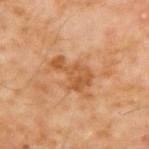<record>
  <biopsy_status>not biopsied; imaged during a skin examination</biopsy_status>
  <lesion_size>
    <long_diameter_mm_approx>5.0</long_diameter_mm_approx>
  </lesion_size>
  <lighting>cross-polarized</lighting>
  <automated_metrics>
    <area_mm2_approx>8.5</area_mm2_approx>
    <eccentricity>0.9</eccentricity>
    <shape_asymmetry>0.6</shape_asymmetry>
    <cielab_L>51</cielab_L>
    <cielab_a>23</cielab_a>
    <cielab_b>39</cielab_b>
    <vs_skin_darker_L>9.0</vs_skin_darker_L>
    <vs_skin_contrast_norm>7.0</vs_skin_contrast_norm>
    <border_irregularity_0_10>7.0</border_irregularity_0_10>
    <color_variation_0_10>3.0</color_variation_0_10>
    <peripheral_color_asymmetry>1.0</peripheral_color_asymmetry>
  </automated_metrics>
  <patient>
    <sex>male</sex>
    <age_approx>60</age_approx>
  </patient>
  <site>upper back</site>
  <image>
    <source>total-body photography crop</source>
    <field_of_view_mm>15</field_of_view_mm>
  </image>
</record>